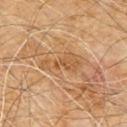Part of a total-body skin-imaging series; this lesion was reviewed on a skin check and was not flagged for biopsy. A 15 mm close-up tile from a total-body photography series done for melanoma screening. Imaged with cross-polarized lighting. From the chest. The lesion-visualizer software estimated a shape eccentricity near 0.9 and a shape-asymmetry score of about 0.45 (0 = symmetric). And it measured a mean CIELAB color near L≈53 a*≈20 b*≈37, a lesion–skin lightness drop of about 8, and a normalized lesion–skin contrast near 5.5. The analysis additionally found border irregularity of about 6 on a 0–10 scale. The software also gave an automated nevus-likeness rating near 0 out of 100 and a detector confidence of about 90 out of 100 that the crop contains a lesion. A male subject, aged around 60.